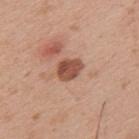Captured during whole-body skin photography for melanoma surveillance; the lesion was not biopsied. The lesion's longest dimension is about 3 mm. A 15 mm crop from a total-body photograph taken for skin-cancer surveillance. The tile uses white-light illumination. On the upper back. A male subject about 30 years old.